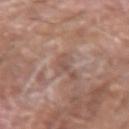This lesion was catalogued during total-body skin photography and was not selected for biopsy.
A region of skin cropped from a whole-body photographic capture, roughly 15 mm wide.
Located on the right forearm.
The patient is a male aged 73 to 77.
Imaged with white-light lighting.